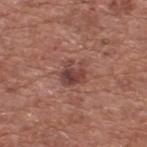{"biopsy_status": "not biopsied; imaged during a skin examination", "patient": {"sex": "male", "age_approx": 75}, "lighting": "white-light", "site": "upper back", "image": {"source": "total-body photography crop", "field_of_view_mm": 15}, "automated_metrics": {"area_mm2_approx": 6.5, "shape_asymmetry": 0.4, "cielab_L": 43, "cielab_a": 23, "cielab_b": 23, "vs_skin_darker_L": 10.0, "vs_skin_contrast_norm": 8.0, "border_irregularity_0_10": 4.5, "peripheral_color_asymmetry": 1.5, "nevus_likeness_0_100": 55, "lesion_detection_confidence_0_100": 100}}